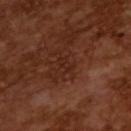workup = catalogued during a skin exam; not biopsied
size = ≈2.5 mm
subject = male, approximately 65 years of age
lighting = cross-polarized illumination
acquisition = ~15 mm tile from a whole-body skin photo